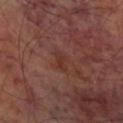follow-up: total-body-photography surveillance lesion; no biopsy | image source: ~15 mm tile from a whole-body skin photo | TBP lesion metrics: a border-irregularity rating of about 3.5/10 and a peripheral color-asymmetry measure near 0.5; an automated nevus-likeness rating near 0 out of 100 and lesion-presence confidence of about 100/100 | tile lighting: cross-polarized | body site: the leg | subject: male, roughly 70 years of age.